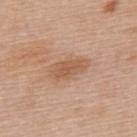On the back. Imaged with white-light lighting. A close-up tile cropped from a whole-body skin photograph, about 15 mm across. The subject is a female roughly 65 years of age.